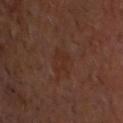  biopsy_status: not biopsied; imaged during a skin examination
  patient:
    sex: male
    age_approx: 60
  site: head or neck
  lesion_size:
    long_diameter_mm_approx: 2.5
  automated_metrics:
    area_mm2_approx: 5.0
    eccentricity: 0.5
    shape_asymmetry: 0.3
    cielab_L: 27
    cielab_a: 19
    cielab_b: 24
    vs_skin_darker_L: 4.0
    vs_skin_contrast_norm: 5.0
    peripheral_color_asymmetry: 0.5
    nevus_likeness_0_100: 0
  image:
    source: total-body photography crop
    field_of_view_mm: 15
  lighting: cross-polarized